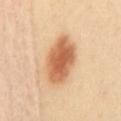<record>
  <biopsy_status>not biopsied; imaged during a skin examination</biopsy_status>
  <lighting>cross-polarized</lighting>
  <site>back</site>
  <patient>
    <sex>female</sex>
    <age_approx>40</age_approx>
  </patient>
  <image>
    <source>total-body photography crop</source>
    <field_of_view_mm>15</field_of_view_mm>
  </image>
  <lesion_size>
    <long_diameter_mm_approx>6.5</long_diameter_mm_approx>
  </lesion_size>
</record>